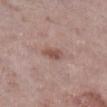location = the right lower leg | automated metrics = a footprint of about 3.5 mm², an eccentricity of roughly 0.8, and a symmetry-axis asymmetry near 0.3; an average lesion color of about L≈51 a*≈20 b*≈23 (CIELAB) and about 10 CIELAB-L* units darker than the surrounding skin; internal color variation of about 1.5 on a 0–10 scale and peripheral color asymmetry of about 0.5; a nevus-likeness score of about 45/100 and a detector confidence of about 100 out of 100 that the crop contains a lesion | subject = male, aged 68–72 | image source = total-body-photography crop, ~15 mm field of view.